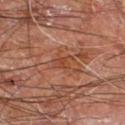Clinical impression: This lesion was catalogued during total-body skin photography and was not selected for biopsy. Image and clinical context: A lesion tile, about 15 mm wide, cut from a 3D total-body photograph. The lesion is located on the right leg. Approximately 2.5 mm at its widest. A male subject, aged approximately 60. Captured under cross-polarized illumination.